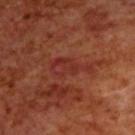Imaged with cross-polarized lighting.
A male subject, about 70 years old.
Longest diameter approximately 5 mm.
On the upper back.
A 15 mm close-up extracted from a 3D total-body photography capture.